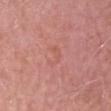Image and clinical context:
This image is a 15 mm lesion crop taken from a total-body photograph. Measured at roughly 2.5 mm in maximum diameter. A male patient, in their mid- to late 80s or older. Located on the head or neck.